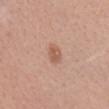biopsy_status: not biopsied; imaged during a skin examination
lesion_size:
  long_diameter_mm_approx: 3.0
lighting: white-light
automated_metrics:
  nevus_likeness_0_100: 65
  lesion_detection_confidence_0_100: 100
site: head or neck
patient:
  sex: female
  age_approx: 45
image:
  source: total-body photography crop
  field_of_view_mm: 15